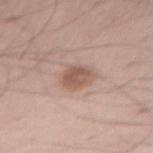Impression: Recorded during total-body skin imaging; not selected for excision or biopsy. Context: About 3 mm across. The total-body-photography lesion software estimated a border-irregularity rating of about 2/10 and internal color variation of about 3 on a 0–10 scale. The analysis additionally found an automated nevus-likeness rating near 90 out of 100 and a detector confidence of about 100 out of 100 that the crop contains a lesion. The subject is a male aged 53 to 57. On the abdomen. Imaged with white-light lighting. A 15 mm crop from a total-body photograph taken for skin-cancer surveillance.